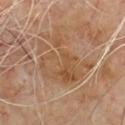  biopsy_status: not biopsied; imaged during a skin examination
  image:
    source: total-body photography crop
    field_of_view_mm: 15
  patient:
    sex: male
    age_approx: 70
  lighting: cross-polarized
  lesion_size:
    long_diameter_mm_approx: 7.0
  site: chest